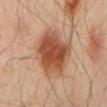Context: A close-up tile cropped from a whole-body skin photograph, about 15 mm across. A male subject, aged 63–67. The lesion is located on the mid back. Measured at roughly 7 mm in maximum diameter. Automated image analysis of the tile measured a lesion area of about 24 mm² and an eccentricity of roughly 0.7. The software also gave roughly 13 lightness units darker than nearby skin and a normalized border contrast of about 9.5. It also reported a border-irregularity index near 2.5/10, a color-variation rating of about 5.5/10, and radial color variation of about 1.5. Captured under cross-polarized illumination.